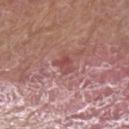Assessment: The lesion was tiled from a total-body skin photograph and was not biopsied. Acquisition and patient details: A roughly 15 mm field-of-view crop from a total-body skin photograph. Imaged with white-light lighting. The subject is a male about 65 years old. On the left upper arm. Measured at roughly 2.5 mm in maximum diameter.